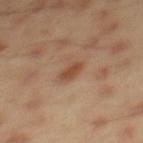{
  "biopsy_status": "not biopsied; imaged during a skin examination",
  "image": {
    "source": "total-body photography crop",
    "field_of_view_mm": 15
  },
  "site": "mid back",
  "lighting": "cross-polarized",
  "patient": {
    "sex": "male",
    "age_approx": 45
  },
  "automated_metrics": {
    "eccentricity": 0.8,
    "shape_asymmetry": 0.25,
    "cielab_L": 47,
    "cielab_a": 22,
    "cielab_b": 32,
    "nevus_likeness_0_100": 75
  }
}